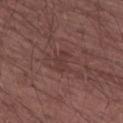Assessment:
No biopsy was performed on this lesion — it was imaged during a full skin examination and was not determined to be concerning.
Context:
A roughly 15 mm field-of-view crop from a total-body skin photograph. A male subject, about 65 years old. Measured at roughly 2.5 mm in maximum diameter. On the right upper arm.